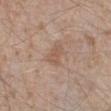Q: Who is the patient?
A: male, roughly 45 years of age
Q: Lesion location?
A: the right lower leg
Q: What lighting was used for the tile?
A: white-light illumination
Q: What kind of image is this?
A: 15 mm crop, total-body photography
Q: How large is the lesion?
A: ~3 mm (longest diameter)